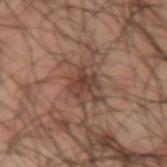Acquisition and patient details: The lesion is located on the left upper arm. Longest diameter approximately 3 mm. The total-body-photography lesion software estimated an area of roughly 5 mm², an outline eccentricity of about 0.65 (0 = round, 1 = elongated), and a shape-asymmetry score of about 0.3 (0 = symmetric). The analysis additionally found a lesion color around L≈29 a*≈15 b*≈19 in CIELAB, roughly 7 lightness units darker than nearby skin, and a normalized border contrast of about 7. It also reported a color-variation rating of about 4/10 and a peripheral color-asymmetry measure near 1.5. The analysis additionally found a nevus-likeness score of about 5/100 and a detector confidence of about 100 out of 100 that the crop contains a lesion. This is a cross-polarized tile. A lesion tile, about 15 mm wide, cut from a 3D total-body photograph. A male subject, in their 50s.